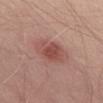Impression: Part of a total-body skin-imaging series; this lesion was reviewed on a skin check and was not flagged for biopsy. Clinical summary: A lesion tile, about 15 mm wide, cut from a 3D total-body photograph. The subject is a male aged 48 to 52. The tile uses white-light illumination. Measured at roughly 4.5 mm in maximum diameter. From the abdomen. An algorithmic analysis of the crop reported a lesion–skin lightness drop of about 10 and a lesion-to-skin contrast of about 7 (normalized; higher = more distinct).